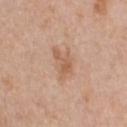follow-up: imaged on a skin check; not biopsied
TBP lesion metrics: a footprint of about 6.5 mm², an eccentricity of roughly 0.8, and a shape-asymmetry score of about 0.45 (0 = symmetric); a border-irregularity rating of about 5/10 and peripheral color asymmetry of about 1
location: the chest
acquisition: total-body-photography crop, ~15 mm field of view
subject: male, aged 58 to 62
diameter: ≈4 mm